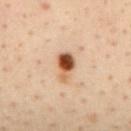{
  "biopsy_status": "not biopsied; imaged during a skin examination",
  "site": "mid back",
  "patient": {
    "sex": "male",
    "age_approx": 45
  },
  "image": {
    "source": "total-body photography crop",
    "field_of_view_mm": 15
  }
}